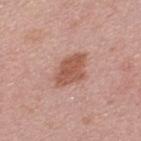{
  "biopsy_status": "not biopsied; imaged during a skin examination",
  "lesion_size": {
    "long_diameter_mm_approx": 4.5
  },
  "patient": {
    "sex": "male",
    "age_approx": 45
  },
  "site": "back",
  "image": {
    "source": "total-body photography crop",
    "field_of_view_mm": 15
  },
  "lighting": "white-light"
}